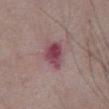{"biopsy_status": "not biopsied; imaged during a skin examination", "image": {"source": "total-body photography crop", "field_of_view_mm": 15}, "automated_metrics": {"border_irregularity_0_10": 2.5, "color_variation_0_10": 6.0, "peripheral_color_asymmetry": 2.0}, "site": "abdomen", "patient": {"sex": "male", "age_approx": "85+"}, "lighting": "white-light"}